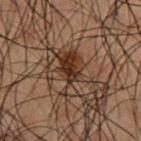biopsy_status: not biopsied; imaged during a skin examination
automated_metrics:
  area_mm2_approx: 8.5
  eccentricity: 0.9
  shape_asymmetry: 0.45
  border_irregularity_0_10: 5.5
  color_variation_0_10: 3.0
  peripheral_color_asymmetry: 1.0
  nevus_likeness_0_100: 95
  lesion_detection_confidence_0_100: 95
lesion_size:
  long_diameter_mm_approx: 5.5
site: front of the torso
patient:
  sex: male
  age_approx: 50
lighting: cross-polarized
image:
  source: total-body photography crop
  field_of_view_mm: 15